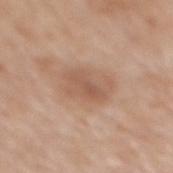The lesion was photographed on a routine skin check and not biopsied; there is no pathology result.
Cropped from a total-body skin-imaging series; the visible field is about 15 mm.
Approximately 4.5 mm at its widest.
The subject is a male in their 70s.
The lesion is on the mid back.
Captured under white-light illumination.